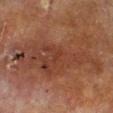The lesion was photographed on a routine skin check and not biopsied; there is no pathology result.
A lesion tile, about 15 mm wide, cut from a 3D total-body photograph.
Captured under cross-polarized illumination.
From the right lower leg.
An algorithmic analysis of the crop reported a lesion area of about 46 mm² and an eccentricity of roughly 0.95. It also reported a lesion color around L≈33 a*≈19 b*≈25 in CIELAB and a normalized lesion–skin contrast near 7. The analysis additionally found a color-variation rating of about 5/10 and a peripheral color-asymmetry measure near 1.5.
A male patient, about 70 years old.